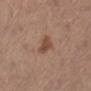The lesion was tiled from a total-body skin photograph and was not biopsied. From the left lower leg. A roughly 15 mm field-of-view crop from a total-body skin photograph. The patient is a female in their mid-60s.